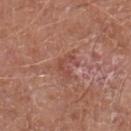<case>
<biopsy_status>not biopsied; imaged during a skin examination</biopsy_status>
</case>